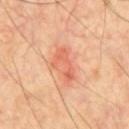Impression: The lesion was photographed on a routine skin check and not biopsied; there is no pathology result. Background: A 15 mm crop from a total-body photograph taken for skin-cancer surveillance. Located on the chest. Automated image analysis of the tile measured a footprint of about 8.5 mm², an eccentricity of roughly 0.85, and a shape-asymmetry score of about 0.4 (0 = symmetric). The analysis additionally found a lesion color around L≈63 a*≈30 b*≈36 in CIELAB and a normalized lesion–skin contrast near 5.5. The software also gave a border-irregularity index near 4.5/10 and peripheral color asymmetry of about 1.5. The analysis additionally found an automated nevus-likeness rating near 10 out of 100 and a lesion-detection confidence of about 100/100. This is a cross-polarized tile. The recorded lesion diameter is about 4.5 mm. A male patient, roughly 60 years of age.